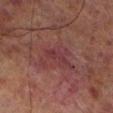The lesion was tiled from a total-body skin photograph and was not biopsied.
A male subject aged 68–72.
Longest diameter approximately 3 mm.
A 15 mm crop from a total-body photograph taken for skin-cancer surveillance.
Captured under cross-polarized illumination.
An algorithmic analysis of the crop reported an area of roughly 4 mm², an eccentricity of roughly 0.9, and a shape-asymmetry score of about 0.4 (0 = symmetric). It also reported radial color variation of about 1.
The lesion is on the leg.